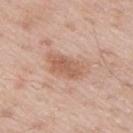* follow-up: catalogued during a skin exam; not biopsied
* anatomic site: the right upper arm
* automated lesion analysis: a lesion area of about 12 mm² and a shape eccentricity near 0.85; a mean CIELAB color near L≈60 a*≈21 b*≈30, about 9 CIELAB-L* units darker than the surrounding skin, and a lesion-to-skin contrast of about 6.5 (normalized; higher = more distinct); border irregularity of about 3 on a 0–10 scale and radial color variation of about 1
* lighting: white-light illumination
* imaging modality: 15 mm crop, total-body photography
* size: ~5 mm (longest diameter)
* subject: male, aged around 65